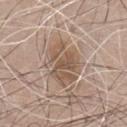Impression:
Captured during whole-body skin photography for melanoma surveillance; the lesion was not biopsied.
Clinical summary:
Imaged with white-light lighting. The lesion's longest dimension is about 5.5 mm. The lesion is on the chest. A region of skin cropped from a whole-body photographic capture, roughly 15 mm wide. The subject is a male aged 63 to 67.